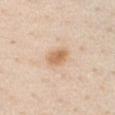Assessment: The lesion was tiled from a total-body skin photograph and was not biopsied. Context: A male patient roughly 60 years of age. Longest diameter approximately 3 mm. The tile uses white-light illumination. On the chest. A 15 mm crop from a total-body photograph taken for skin-cancer surveillance.